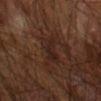Findings:
• biopsy status — catalogued during a skin exam; not biopsied
• patient — male, aged approximately 65
• lesion size — ≈3.5 mm
• body site — the left arm
• tile lighting — cross-polarized
• image source — ~15 mm tile from a whole-body skin photo
• image-analysis metrics — an area of roughly 7.5 mm² and a shape-asymmetry score of about 0.3 (0 = symmetric); a border-irregularity index near 3.5/10 and a peripheral color-asymmetry measure near 1.5; lesion-presence confidence of about 95/100